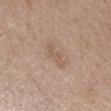| key | value |
|---|---|
| biopsy status | catalogued during a skin exam; not biopsied |
| diameter | about 3 mm |
| imaging modality | total-body-photography crop, ~15 mm field of view |
| lighting | white-light |
| anatomic site | the left forearm |
| image-analysis metrics | a footprint of about 4.5 mm², an outline eccentricity of about 0.85 (0 = round, 1 = elongated), and a symmetry-axis asymmetry near 0.4; a lesion color around L≈57 a*≈16 b*≈28 in CIELAB and roughly 6 lightness units darker than nearby skin; a border-irregularity index near 5.5/10 |
| subject | male, aged 58 to 62 |